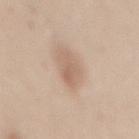Image and clinical context: The tile uses white-light illumination. The lesion is located on the mid back. A female patient about 30 years old. Cropped from a total-body skin-imaging series; the visible field is about 15 mm. The lesion's longest dimension is about 3.5 mm. An algorithmic analysis of the crop reported a footprint of about 6 mm², a shape eccentricity near 0.75, and a shape-asymmetry score of about 0.3 (0 = symmetric). The software also gave a border-irregularity index near 3/10 and a within-lesion color-variation index near 3/10.